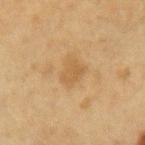The lesion was tiled from a total-body skin photograph and was not biopsied.